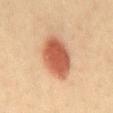Assessment: Imaged during a routine full-body skin examination; the lesion was not biopsied and no histopathology is available.